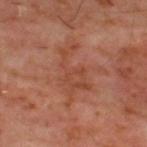Measured at roughly 6 mm in maximum diameter. From the upper back. A close-up tile cropped from a whole-body skin photograph, about 15 mm across. A male subject, about 60 years old. This is a cross-polarized tile.